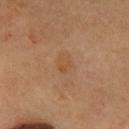Q: Was this lesion biopsied?
A: imaged on a skin check; not biopsied
Q: What is the anatomic site?
A: the front of the torso
Q: Who is the patient?
A: male, approximately 55 years of age
Q: How was this image acquired?
A: ~15 mm crop, total-body skin-cancer survey